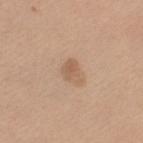Part of a total-body skin-imaging series; this lesion was reviewed on a skin check and was not flagged for biopsy.
The total-body-photography lesion software estimated a border-irregularity rating of about 3/10, a color-variation rating of about 3/10, and a peripheral color-asymmetry measure near 1.
The lesion is on the left thigh.
A 15 mm close-up extracted from a 3D total-body photography capture.
About 2.5 mm across.
A female patient aged approximately 60.
The tile uses white-light illumination.